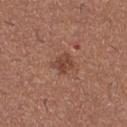Assessment: The lesion was photographed on a routine skin check and not biopsied; there is no pathology result. Clinical summary: Cropped from a whole-body photographic skin survey; the tile spans about 15 mm. This is a white-light tile. On the leg. An algorithmic analysis of the crop reported an area of roughly 4.5 mm², an eccentricity of roughly 0.45, and two-axis asymmetry of about 0.25. The analysis additionally found a mean CIELAB color near L≈44 a*≈23 b*≈27 and a lesion–skin lightness drop of about 9. The subject is a female approximately 25 years of age. Measured at roughly 2.5 mm in maximum diameter.